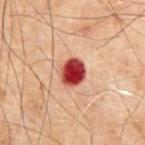Clinical summary: About 3 mm across. A male subject aged around 70. A 15 mm close-up extracted from a 3D total-body photography capture. An algorithmic analysis of the crop reported a footprint of about 7.5 mm², an outline eccentricity of about 0.4 (0 = round, 1 = elongated), and two-axis asymmetry of about 0.15. And it measured about 19 CIELAB-L* units darker than the surrounding skin and a lesion-to-skin contrast of about 15 (normalized; higher = more distinct). And it measured border irregularity of about 1.5 on a 0–10 scale, a within-lesion color-variation index near 6/10, and a peripheral color-asymmetry measure near 1.5. It also reported an automated nevus-likeness rating near 0 out of 100. The lesion is located on the abdomen. Captured under cross-polarized illumination.